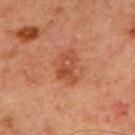Q: Is there a histopathology result?
A: total-body-photography surveillance lesion; no biopsy
Q: Where on the body is the lesion?
A: the chest
Q: What did automated image analysis measure?
A: an area of roughly 8.5 mm², an outline eccentricity of about 0.8 (0 = round, 1 = elongated), and two-axis asymmetry of about 0.3; an average lesion color of about L≈39 a*≈23 b*≈29 (CIELAB), a lesion–skin lightness drop of about 7, and a lesion-to-skin contrast of about 6.5 (normalized; higher = more distinct); a border-irregularity index near 3.5/10, a within-lesion color-variation index near 4/10, and radial color variation of about 1.5; a classifier nevus-likeness of about 15/100 and lesion-presence confidence of about 100/100
Q: How large is the lesion?
A: ≈4 mm
Q: Who is the patient?
A: male, aged around 65
Q: What is the imaging modality?
A: ~15 mm tile from a whole-body skin photo